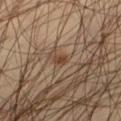Notes:
• notes — total-body-photography surveillance lesion; no biopsy
• diameter — ≈2 mm
• patient — male, aged 43–47
• lighting — cross-polarized
• image — total-body-photography crop, ~15 mm field of view
• location — the left thigh
• image-analysis metrics — an average lesion color of about L≈41 a*≈18 b*≈29 (CIELAB) and a lesion-to-skin contrast of about 7.5 (normalized; higher = more distinct)